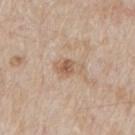* workup: no biopsy performed (imaged during a skin exam)
* lighting: white-light
* automated lesion analysis: a footprint of about 5 mm², an eccentricity of roughly 0.8, and a symmetry-axis asymmetry near 0.3; an average lesion color of about L≈59 a*≈16 b*≈30 (CIELAB) and about 9 CIELAB-L* units darker than the surrounding skin; a border-irregularity index near 2.5/10, a color-variation rating of about 5/10, and radial color variation of about 1.5
* patient: male, aged around 65
* acquisition: 15 mm crop, total-body photography
* anatomic site: the mid back
* size: ~3.5 mm (longest diameter)